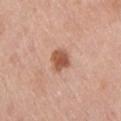lesion_size:
  long_diameter_mm_approx: 2.5
image:
  source: total-body photography crop
  field_of_view_mm: 15
automated_metrics:
  cielab_L: 55
  cielab_a: 24
  cielab_b: 32
  vs_skin_darker_L: 13.0
  vs_skin_contrast_norm: 9.0
  nevus_likeness_0_100: 90
  lesion_detection_confidence_0_100: 100
site: upper back
lighting: white-light
patient:
  sex: male
  age_approx: 45